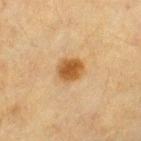| key | value |
|---|---|
| workup | catalogued during a skin exam; not biopsied |
| subject | female, aged 53 to 57 |
| size | about 3 mm |
| automated metrics | a mean CIELAB color near L≈47 a*≈18 b*≈37 and roughly 12 lightness units darker than nearby skin; lesion-presence confidence of about 100/100 |
| location | the left thigh |
| image source | total-body-photography crop, ~15 mm field of view |